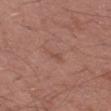<tbp_lesion>
  <biopsy_status>not biopsied; imaged during a skin examination</biopsy_status>
</tbp_lesion>